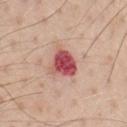notes = catalogued during a skin exam; not biopsied
subject = male, in their 40s
location = the chest
illumination = white-light
image source = ~15 mm tile from a whole-body skin photo
lesion diameter = ≈3.5 mm